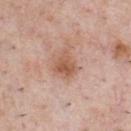biopsy status: no biopsy performed (imaged during a skin exam)
image: ~15 mm tile from a whole-body skin photo
site: the chest
patient: male, aged around 40